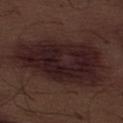Clinical impression: Recorded during total-body skin imaging; not selected for excision or biopsy. Context: A close-up tile cropped from a whole-body skin photograph, about 15 mm across. The lesion is located on the abdomen. The patient is a male about 70 years old. About 12 mm across. Captured under white-light illumination. The lesion-visualizer software estimated a mean CIELAB color near L≈20 a*≈17 b*≈15, roughly 9 lightness units darker than nearby skin, and a lesion-to-skin contrast of about 12 (normalized; higher = more distinct). It also reported a color-variation rating of about 4.5/10. It also reported a classifier nevus-likeness of about 55/100.